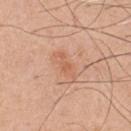  biopsy_status: not biopsied; imaged during a skin examination
  site: chest
  patient:
    sex: male
    age_approx: 50
  image:
    source: total-body photography crop
    field_of_view_mm: 15